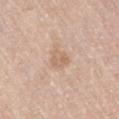The lesion was tiled from a total-body skin photograph and was not biopsied. The tile uses white-light illumination. The patient is a male in their 80s. A 15 mm crop from a total-body photograph taken for skin-cancer surveillance. Located on the right thigh. About 2.5 mm across.